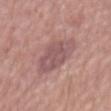Acquisition and patient details:
A male subject aged around 65. The recorded lesion diameter is about 6.5 mm. A region of skin cropped from a whole-body photographic capture, roughly 15 mm wide. This is a white-light tile. Located on the mid back.